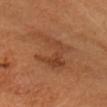Part of a total-body skin-imaging series; this lesion was reviewed on a skin check and was not flagged for biopsy. The lesion is on the head or neck. About 6.5 mm across. A female patient, aged 58–62. A 15 mm close-up extracted from a 3D total-body photography capture.